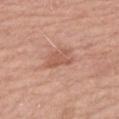Q: Was a biopsy performed?
A: imaged on a skin check; not biopsied
Q: What is the imaging modality?
A: total-body-photography crop, ~15 mm field of view
Q: What did automated image analysis measure?
A: a footprint of about 5.5 mm²; a lesion color around L≈56 a*≈24 b*≈29 in CIELAB, about 9 CIELAB-L* units darker than the surrounding skin, and a normalized border contrast of about 6; a classifier nevus-likeness of about 15/100 and lesion-presence confidence of about 100/100
Q: Illumination type?
A: white-light illumination
Q: Where on the body is the lesion?
A: the arm
Q: Who is the patient?
A: female, aged around 65
Q: How large is the lesion?
A: ≈3 mm